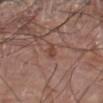Part of a total-body skin-imaging series; this lesion was reviewed on a skin check and was not flagged for biopsy. An algorithmic analysis of the crop reported an eccentricity of roughly 0.95 and a symmetry-axis asymmetry near 0.3. The software also gave a lesion color around L≈44 a*≈22 b*≈27 in CIELAB, a lesion–skin lightness drop of about 8, and a normalized border contrast of about 7. The software also gave a border-irregularity rating of about 3.5/10 and radial color variation of about 0. The analysis additionally found a nevus-likeness score of about 0/100 and a detector confidence of about 90 out of 100 that the crop contains a lesion. Measured at roughly 2.5 mm in maximum diameter. From the right arm. This image is a 15 mm lesion crop taken from a total-body photograph. A male patient aged 78 to 82. Imaged with white-light lighting.